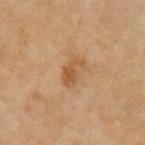Imaged during a routine full-body skin examination; the lesion was not biopsied and no histopathology is available. A lesion tile, about 15 mm wide, cut from a 3D total-body photograph. The lesion's longest dimension is about 3.5 mm. From the left upper arm. A female subject in their 60s. Imaged with cross-polarized lighting.